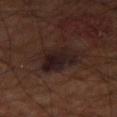Q: Is there a histopathology result?
A: no biopsy performed (imaged during a skin exam)
Q: Lesion location?
A: the right thigh
Q: What is the imaging modality?
A: ~15 mm crop, total-body skin-cancer survey
Q: How large is the lesion?
A: about 5 mm
Q: How was the tile lit?
A: cross-polarized
Q: What are the patient's age and sex?
A: male, aged 68–72